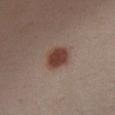Case summary:
* image source — ~15 mm crop, total-body skin-cancer survey
* patient — female, approximately 30 years of age
* illumination — white-light
* image-analysis metrics — an eccentricity of roughly 0.6 and two-axis asymmetry of about 0.2; a border-irregularity rating of about 1.5/10, internal color variation of about 2.5 on a 0–10 scale, and radial color variation of about 0.5
* lesion size — about 3.5 mm
* body site — the chest